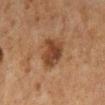| key | value |
|---|---|
| workup | total-body-photography surveillance lesion; no biopsy |
| acquisition | ~15 mm tile from a whole-body skin photo |
| location | the right lower leg |
| automated metrics | a footprint of about 11 mm², an eccentricity of roughly 0.3, and two-axis asymmetry of about 0.25; a border-irregularity rating of about 2.5/10, a within-lesion color-variation index near 3.5/10, and peripheral color asymmetry of about 1; lesion-presence confidence of about 100/100 |
| lighting | cross-polarized |
| subject | male, aged approximately 65 |
| size | ≈4 mm |